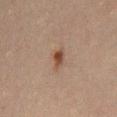Impression: The lesion was tiled from a total-body skin photograph and was not biopsied. Clinical summary: Automated image analysis of the tile measured an area of roughly 3.5 mm² and an eccentricity of roughly 0.8. The software also gave a border-irregularity index near 2/10, a color-variation rating of about 3/10, and radial color variation of about 1. The analysis additionally found a classifier nevus-likeness of about 90/100 and a lesion-detection confidence of about 100/100. This image is a 15 mm lesion crop taken from a total-body photograph. A male subject, approximately 45 years of age. On the front of the torso. Imaged with cross-polarized lighting. Measured at roughly 2.5 mm in maximum diameter.